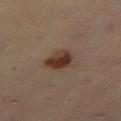Clinical impression:
Captured during whole-body skin photography for melanoma surveillance; the lesion was not biopsied.
Clinical summary:
Cropped from a whole-body photographic skin survey; the tile spans about 15 mm. From the leg. The subject is a female aged approximately 35.